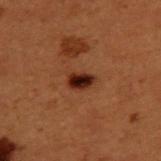notes: imaged on a skin check; not biopsied
illumination: cross-polarized illumination
lesion diameter: about 3 mm
anatomic site: the upper back
automated metrics: a border-irregularity rating of about 2.5/10, a color-variation rating of about 5/10, and radial color variation of about 1.5
subject: male, in their 50s
image source: ~15 mm crop, total-body skin-cancer survey